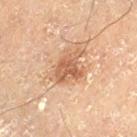workup — total-body-photography surveillance lesion; no biopsy | subject — male, aged approximately 70 | image-analysis metrics — a lesion area of about 8 mm², an outline eccentricity of about 0.65 (0 = round, 1 = elongated), and two-axis asymmetry of about 0.45; a lesion-to-skin contrast of about 7.5 (normalized; higher = more distinct) | location — the left thigh | lesion diameter — ~4 mm (longest diameter) | image source — ~15 mm tile from a whole-body skin photo.